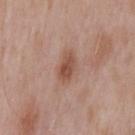Impression:
Recorded during total-body skin imaging; not selected for excision or biopsy.
Clinical summary:
A male subject aged 53 to 57. A region of skin cropped from a whole-body photographic capture, roughly 15 mm wide. The lesion is on the mid back. Captured under white-light illumination.